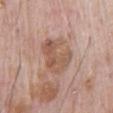The lesion was tiled from a total-body skin photograph and was not biopsied. A male subject, roughly 70 years of age. The lesion is located on the chest. A 15 mm close-up extracted from a 3D total-body photography capture.